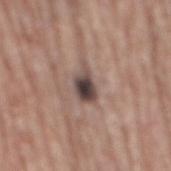notes — total-body-photography surveillance lesion; no biopsy
site — the mid back
lesion size — ~4.5 mm (longest diameter)
patient — male, aged approximately 75
automated lesion analysis — a lesion area of about 6.5 mm², an eccentricity of roughly 0.85, and a symmetry-axis asymmetry near 0.35; a border-irregularity rating of about 4/10 and a within-lesion color-variation index near 8/10
lighting — white-light illumination
imaging modality — ~15 mm crop, total-body skin-cancer survey